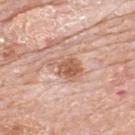Q: Was this lesion biopsied?
A: imaged on a skin check; not biopsied
Q: Patient demographics?
A: male, approximately 80 years of age
Q: What is the lesion's diameter?
A: ~4 mm (longest diameter)
Q: How was this image acquired?
A: 15 mm crop, total-body photography
Q: Lesion location?
A: the upper back
Q: Automated lesion metrics?
A: border irregularity of about 3.5 on a 0–10 scale, a color-variation rating of about 4.5/10, and radial color variation of about 1.5; lesion-presence confidence of about 100/100